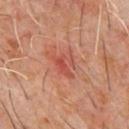Assessment:
Part of a total-body skin-imaging series; this lesion was reviewed on a skin check and was not flagged for biopsy.
Clinical summary:
A male patient, aged approximately 60. A close-up tile cropped from a whole-body skin photograph, about 15 mm across. Located on the chest.